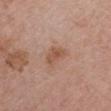Assessment: The lesion was tiled from a total-body skin photograph and was not biopsied.